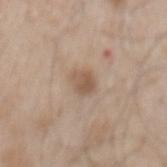site: back
patient:
  sex: male
  age_approx: 50
lesion_size:
  long_diameter_mm_approx: 2.5
image:
  source: total-body photography crop
  field_of_view_mm: 15